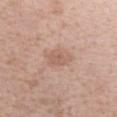| field | value |
|---|---|
| biopsy status | catalogued during a skin exam; not biopsied |
| imaging modality | ~15 mm crop, total-body skin-cancer survey |
| tile lighting | white-light illumination |
| location | the right forearm |
| size | ≈3.5 mm |
| automated lesion analysis | a lesion color around L≈60 a*≈19 b*≈27 in CIELAB, roughly 8 lightness units darker than nearby skin, and a lesion-to-skin contrast of about 5 (normalized; higher = more distinct); a within-lesion color-variation index near 2/10 and peripheral color asymmetry of about 0.5 |
| patient | female, approximately 40 years of age |